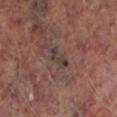Context: The lesion is on the left lower leg. About 3 mm across. A 15 mm close-up tile from a total-body photography series done for melanoma screening. The patient is a male aged approximately 65. Automated image analysis of the tile measured a shape eccentricity near 0.9 and a symmetry-axis asymmetry near 0.55. And it measured a nevus-likeness score of about 0/100 and a detector confidence of about 80 out of 100 that the crop contains a lesion. This is a cross-polarized tile.